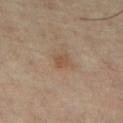Q: Is there a histopathology result?
A: no biopsy performed (imaged during a skin exam)
Q: What did automated image analysis measure?
A: an outline eccentricity of about 0.65 (0 = round, 1 = elongated); an average lesion color of about L≈51 a*≈18 b*≈31 (CIELAB), about 7 CIELAB-L* units darker than the surrounding skin, and a normalized lesion–skin contrast near 6; a border-irregularity index near 3/10, internal color variation of about 0.5 on a 0–10 scale, and radial color variation of about 0
Q: What are the patient's age and sex?
A: female, aged 63–67
Q: What kind of image is this?
A: total-body-photography crop, ~15 mm field of view
Q: Lesion location?
A: the right lower leg